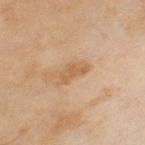Findings:
– workup · no biopsy performed (imaged during a skin exam)
– acquisition · 15 mm crop, total-body photography
– patient · female, aged 58 to 62
– body site · the left thigh